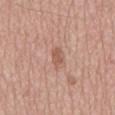Imaged during a routine full-body skin examination; the lesion was not biopsied and no histopathology is available. A 15 mm close-up extracted from a 3D total-body photography capture. Approximately 2.5 mm at its widest. Captured under white-light illumination. The subject is a male aged approximately 65. The lesion is on the back.